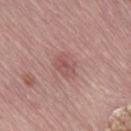Recorded during total-body skin imaging; not selected for excision or biopsy.
A female patient aged 63 to 67.
The total-body-photography lesion software estimated a mean CIELAB color near L≈53 a*≈24 b*≈22, about 8 CIELAB-L* units darker than the surrounding skin, and a normalized border contrast of about 5.5. The software also gave a color-variation rating of about 3/10 and a peripheral color-asymmetry measure near 1.
The tile uses white-light illumination.
The recorded lesion diameter is about 2.5 mm.
The lesion is on the right thigh.
A 15 mm close-up extracted from a 3D total-body photography capture.